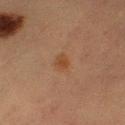Q: Was a biopsy performed?
A: total-body-photography surveillance lesion; no biopsy
Q: What is the imaging modality?
A: ~15 mm crop, total-body skin-cancer survey
Q: Illumination type?
A: cross-polarized illumination
Q: Where on the body is the lesion?
A: the leg
Q: How large is the lesion?
A: about 2.5 mm
Q: Patient demographics?
A: female, in their mid- to late 50s